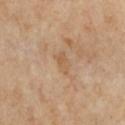biopsy status — total-body-photography surveillance lesion; no biopsy | tile lighting — cross-polarized | subject — female, aged 68 to 72 | imaging modality — 15 mm crop, total-body photography | size — ≈3 mm | location — the chest.